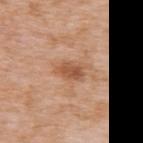The lesion was tiled from a total-body skin photograph and was not biopsied.
From the upper back.
A roughly 15 mm field-of-view crop from a total-body skin photograph.
This is a white-light tile.
A female subject, approximately 40 years of age.
The total-body-photography lesion software estimated a lesion area of about 5.5 mm². The software also gave a border-irregularity index near 2/10, a within-lesion color-variation index near 3/10, and peripheral color asymmetry of about 1.
Measured at roughly 3.5 mm in maximum diameter.